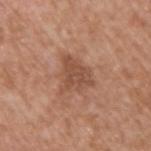workup: total-body-photography surveillance lesion; no biopsy
size: ≈4 mm
anatomic site: the upper back
subject: male, aged 63–67
imaging modality: total-body-photography crop, ~15 mm field of view
illumination: white-light